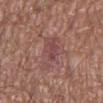notes: total-body-photography surveillance lesion; no biopsy | subject: male, aged approximately 55 | size: ≈6 mm | anatomic site: the left lower leg | illumination: white-light | automated lesion analysis: a footprint of about 11 mm² and a symmetry-axis asymmetry near 0.35; an average lesion color of about L≈47 a*≈22 b*≈21 (CIELAB), a lesion–skin lightness drop of about 7, and a lesion-to-skin contrast of about 6 (normalized; higher = more distinct); a classifier nevus-likeness of about 0/100 and a lesion-detection confidence of about 85/100 | image source: ~15 mm tile from a whole-body skin photo.